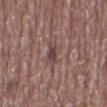Q: Is there a histopathology result?
A: imaged on a skin check; not biopsied
Q: What lighting was used for the tile?
A: white-light
Q: Where on the body is the lesion?
A: the mid back
Q: What are the patient's age and sex?
A: male, approximately 75 years of age
Q: How was this image acquired?
A: ~15 mm tile from a whole-body skin photo
Q: What did automated image analysis measure?
A: an automated nevus-likeness rating near 0 out of 100
Q: Lesion size?
A: ≈3.5 mm